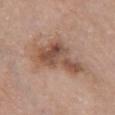Impression: Recorded during total-body skin imaging; not selected for excision or biopsy. Clinical summary: From the chest. The lesion's longest dimension is about 7.5 mm. A female subject aged around 65. A close-up tile cropped from a whole-body skin photograph, about 15 mm across. Captured under white-light illumination.